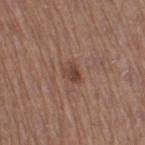On the leg. Imaged with white-light lighting. Cropped from a total-body skin-imaging series; the visible field is about 15 mm. Longest diameter approximately 2.5 mm. A female patient about 55 years old.